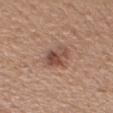Imaged during a routine full-body skin examination; the lesion was not biopsied and no histopathology is available.